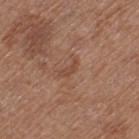| feature | finding |
|---|---|
| workup | catalogued during a skin exam; not biopsied |
| tile lighting | white-light illumination |
| automated lesion analysis | a footprint of about 2.5 mm² and a symmetry-axis asymmetry near 0.45; internal color variation of about 0 on a 0–10 scale and radial color variation of about 0; lesion-presence confidence of about 100/100 |
| acquisition | ~15 mm tile from a whole-body skin photo |
| location | the leg |
| subject | female, approximately 40 years of age |